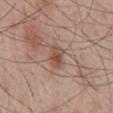Case summary:
- follow-up — total-body-photography surveillance lesion; no biopsy
- image source — 15 mm crop, total-body photography
- lighting — white-light
- diameter — ~3 mm (longest diameter)
- location — the mid back
- patient — male, in their mid- to late 50s